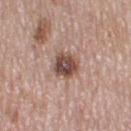notes: catalogued during a skin exam; not biopsied
lesion size: ~3.5 mm (longest diameter)
location: the leg
subject: female, aged around 50
imaging modality: ~15 mm tile from a whole-body skin photo